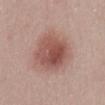{"automated_metrics": {"border_irregularity_0_10": 1.0, "color_variation_0_10": 5.5, "peripheral_color_asymmetry": 2.0, "nevus_likeness_0_100": 95, "lesion_detection_confidence_0_100": 100}, "site": "front of the torso", "image": {"source": "total-body photography crop", "field_of_view_mm": 15}, "lighting": "white-light", "lesion_size": {"long_diameter_mm_approx": 5.0}, "patient": {"sex": "female", "age_approx": 30}}